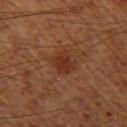Q: Was this lesion biopsied?
A: no biopsy performed (imaged during a skin exam)
Q: What is the anatomic site?
A: the leg
Q: What kind of image is this?
A: total-body-photography crop, ~15 mm field of view
Q: Illumination type?
A: cross-polarized
Q: How large is the lesion?
A: ≈3 mm
Q: Who is the patient?
A: male, roughly 55 years of age